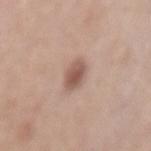Part of a total-body skin-imaging series; this lesion was reviewed on a skin check and was not flagged for biopsy. This is a white-light tile. A male subject, about 60 years old. The lesion's longest dimension is about 3 mm. From the mid back. Automated image analysis of the tile measured an area of roughly 5 mm², a shape eccentricity near 0.7, and two-axis asymmetry of about 0.2. It also reported a mean CIELAB color near L≈54 a*≈20 b*≈25, about 13 CIELAB-L* units darker than the surrounding skin, and a normalized lesion–skin contrast near 8.5. It also reported border irregularity of about 1.5 on a 0–10 scale, a color-variation rating of about 3/10, and radial color variation of about 1. A region of skin cropped from a whole-body photographic capture, roughly 15 mm wide.